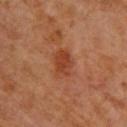{
  "lesion_size": {
    "long_diameter_mm_approx": 3.5
  },
  "site": "upper back",
  "patient": {
    "sex": "female",
    "age_approx": 65
  },
  "image": {
    "source": "total-body photography crop",
    "field_of_view_mm": 15
  }
}